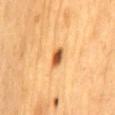biopsy status — total-body-photography surveillance lesion; no biopsy | tile lighting — cross-polarized illumination | diameter — ~3 mm (longest diameter) | body site — the mid back | image source — ~15 mm tile from a whole-body skin photo | patient — male, aged around 60.